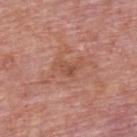The lesion is on the back. The recorded lesion diameter is about 3.5 mm. An algorithmic analysis of the crop reported an area of roughly 4.5 mm², a shape eccentricity near 0.8, and two-axis asymmetry of about 0.5. It also reported a border-irregularity rating of about 5/10, a color-variation rating of about 3/10, and peripheral color asymmetry of about 1. The analysis additionally found a classifier nevus-likeness of about 0/100 and a lesion-detection confidence of about 100/100. A male subject, approximately 75 years of age. A roughly 15 mm field-of-view crop from a total-body skin photograph.